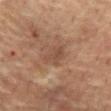Findings:
* lighting: cross-polarized
* imaging modality: ~15 mm crop, total-body skin-cancer survey
* anatomic site: the front of the torso
* subject: male, approximately 65 years of age
* image-analysis metrics: a symmetry-axis asymmetry near 0.4; a border-irregularity rating of about 4/10, a color-variation rating of about 1/10, and a peripheral color-asymmetry measure near 0.5